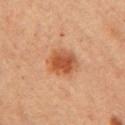Assessment:
The lesion was tiled from a total-body skin photograph and was not biopsied.
Acquisition and patient details:
From the right upper arm. A close-up tile cropped from a whole-body skin photograph, about 15 mm across. A female patient in their 60s.